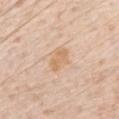Assessment: Recorded during total-body skin imaging; not selected for excision or biopsy. Background: The patient is a male aged around 70. Located on the front of the torso. The lesion's longest dimension is about 3.5 mm. A lesion tile, about 15 mm wide, cut from a 3D total-body photograph.